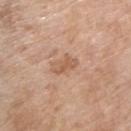{"image": {"source": "total-body photography crop", "field_of_view_mm": 15}, "automated_metrics": {"cielab_L": 58, "cielab_a": 21, "cielab_b": 32, "vs_skin_contrast_norm": 6.0}, "site": "arm", "lighting": "white-light", "patient": {"sex": "female", "age_approx": 75}}